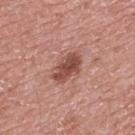| field | value |
|---|---|
| biopsy status | catalogued during a skin exam; not biopsied |
| TBP lesion metrics | an average lesion color of about L≈49 a*≈25 b*≈27 (CIELAB) and a normalized lesion–skin contrast near 9 |
| anatomic site | the back |
| image source | ~15 mm crop, total-body skin-cancer survey |
| subject | male, aged approximately 70 |
| illumination | white-light |